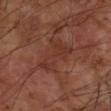Imaged during a routine full-body skin examination; the lesion was not biopsied and no histopathology is available.
Located on the arm.
A lesion tile, about 15 mm wide, cut from a 3D total-body photograph.
Automated tile analysis of the lesion measured a shape eccentricity near 0.8 and a shape-asymmetry score of about 0.45 (0 = symmetric).
The subject is a male aged 68–72.
Longest diameter approximately 5.5 mm.